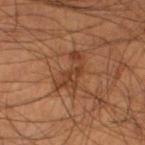<record>
<biopsy_status>not biopsied; imaged during a skin examination</biopsy_status>
<image>
  <source>total-body photography crop</source>
  <field_of_view_mm>15</field_of_view_mm>
</image>
<site>left lower leg</site>
<lesion_size>
  <long_diameter_mm_approx>4.5</long_diameter_mm_approx>
</lesion_size>
<patient>
  <sex>male</sex>
  <age_approx>55</age_approx>
</patient>
</record>